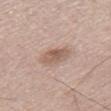<lesion>
<biopsy_status>not biopsied; imaged during a skin examination</biopsy_status>
<automated_metrics>
  <area_mm2_approx>6.0</area_mm2_approx>
  <eccentricity>0.75</eccentricity>
  <shape_asymmetry>0.2</shape_asymmetry>
  <cielab_L>57</cielab_L>
  <cielab_a>17</cielab_a>
  <cielab_b>26</cielab_b>
  <vs_skin_contrast_norm>7.0</vs_skin_contrast_norm>
  <border_irregularity_0_10>2.0</border_irregularity_0_10>
  <peripheral_color_asymmetry>1.0</peripheral_color_asymmetry>
</automated_metrics>
<lighting>white-light</lighting>
<site>right thigh</site>
<image>
  <source>total-body photography crop</source>
  <field_of_view_mm>15</field_of_view_mm>
</image>
<lesion_size>
  <long_diameter_mm_approx>3.5</long_diameter_mm_approx>
</lesion_size>
<patient>
  <sex>male</sex>
  <age_approx>60</age_approx>
</patient>
</lesion>